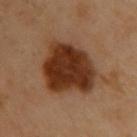Captured during whole-body skin photography for melanoma surveillance; the lesion was not biopsied. The subject is a female aged 58 to 62. A region of skin cropped from a whole-body photographic capture, roughly 15 mm wide. Measured at roughly 7 mm in maximum diameter. The lesion is on the upper back.